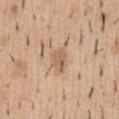lesion size: ~3 mm (longest diameter)
location: the abdomen
image-analysis metrics: an average lesion color of about L≈60 a*≈18 b*≈33 (CIELAB), a lesion–skin lightness drop of about 9, and a lesion-to-skin contrast of about 6.5 (normalized; higher = more distinct); border irregularity of about 3 on a 0–10 scale, a color-variation rating of about 2.5/10, and a peripheral color-asymmetry measure near 1; a detector confidence of about 100 out of 100 that the crop contains a lesion
patient: male, in their 40s
illumination: white-light illumination
acquisition: total-body-photography crop, ~15 mm field of view